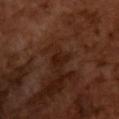{
  "biopsy_status": "not biopsied; imaged during a skin examination",
  "patient": {
    "sex": "male",
    "age_approx": 65
  },
  "lighting": "cross-polarized",
  "automated_metrics": {
    "cielab_L": 19,
    "cielab_a": 19,
    "cielab_b": 23,
    "vs_skin_darker_L": 6.0,
    "color_variation_0_10": 1.0
  },
  "lesion_size": {
    "long_diameter_mm_approx": 2.5
  },
  "image": {
    "source": "total-body photography crop",
    "field_of_view_mm": 15
  }
}